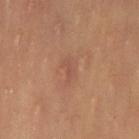{
  "lesion_size": {
    "long_diameter_mm_approx": 2.5
  },
  "image": {
    "source": "total-body photography crop",
    "field_of_view_mm": 15
  },
  "patient": {
    "sex": "female",
    "age_approx": 35
  },
  "automated_metrics": {
    "eccentricity": 0.9,
    "shape_asymmetry": 0.5,
    "cielab_L": 51,
    "cielab_a": 22,
    "cielab_b": 29,
    "vs_skin_contrast_norm": 4.5,
    "border_irregularity_0_10": 5.5,
    "color_variation_0_10": 0.0,
    "peripheral_color_asymmetry": 0.0,
    "nevus_likeness_0_100": 0,
    "lesion_detection_confidence_0_100": 100
  },
  "lighting": "cross-polarized",
  "site": "left thigh"
}